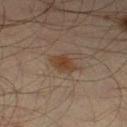Imaged during a routine full-body skin examination; the lesion was not biopsied and no histopathology is available. Automated image analysis of the tile measured an average lesion color of about L≈37 a*≈15 b*≈26 (CIELAB), about 7 CIELAB-L* units darker than the surrounding skin, and a normalized lesion–skin contrast near 8. It also reported border irregularity of about 2.5 on a 0–10 scale, a within-lesion color-variation index near 2/10, and radial color variation of about 0.5. The software also gave a nevus-likeness score of about 85/100 and a lesion-detection confidence of about 100/100. A male subject aged around 35. A lesion tile, about 15 mm wide, cut from a 3D total-body photograph. The recorded lesion diameter is about 3 mm. The tile uses cross-polarized illumination. Located on the left thigh.